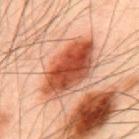Recorded during total-body skin imaging; not selected for excision or biopsy. Captured under cross-polarized illumination. Automated image analysis of the tile measured a footprint of about 24 mm², a shape eccentricity near 0.85, and a shape-asymmetry score of about 0.15 (0 = symmetric). The analysis additionally found an automated nevus-likeness rating near 50 out of 100 and a detector confidence of about 100 out of 100 that the crop contains a lesion. A male patient, aged 48 to 52. The lesion is on the upper back. A 15 mm crop from a total-body photograph taken for skin-cancer surveillance. The recorded lesion diameter is about 8 mm.